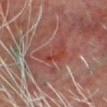A close-up tile cropped from a whole-body skin photograph, about 15 mm across.
A male subject aged around 65.
The lesion is located on the head or neck.
Captured under cross-polarized illumination.
Automated tile analysis of the lesion measured about 6 CIELAB-L* units darker than the surrounding skin and a normalized lesion–skin contrast near 6.5. It also reported a nevus-likeness score of about 0/100 and lesion-presence confidence of about 5/100.
Histopathology of the biopsied lesion showed a nodular basal cell carcinoma — a skin cancer.